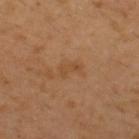Impression:
This lesion was catalogued during total-body skin photography and was not selected for biopsy.
Context:
Captured under cross-polarized illumination. On the upper back. About 2.5 mm across. A male patient, about 30 years old. A 15 mm crop from a total-body photograph taken for skin-cancer surveillance.